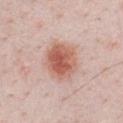A male patient, in their mid-20s.
The lesion is located on the chest.
A 15 mm close-up extracted from a 3D total-body photography capture.